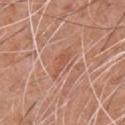lesion size = ~3.5 mm (longest diameter) | body site = the chest | image source = ~15 mm tile from a whole-body skin photo | lighting = white-light | TBP lesion metrics = a border-irregularity rating of about 5.5/10 and peripheral color asymmetry of about 0; an automated nevus-likeness rating near 0 out of 100 | patient = male, in their 60s.